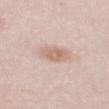notes = total-body-photography surveillance lesion; no biopsy
acquisition = ~15 mm tile from a whole-body skin photo
subject = male, in their mid- to late 20s
anatomic site = the back
image-analysis metrics = a lesion area of about 6.5 mm² and a shape eccentricity near 0.75; an average lesion color of about L≈66 a*≈19 b*≈26 (CIELAB), roughly 9 lightness units darker than nearby skin, and a normalized border contrast of about 6; a within-lesion color-variation index near 2.5/10 and a peripheral color-asymmetry measure near 1